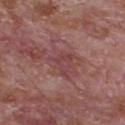  biopsy_status: not biopsied; imaged during a skin examination
  lighting: white-light
  image:
    source: total-body photography crop
    field_of_view_mm: 15
  lesion_size:
    long_diameter_mm_approx: 3.0
  automated_metrics:
    nevus_likeness_0_100: 0
  site: chest
  patient:
    sex: male
    age_approx: 65